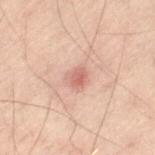| key | value |
|---|---|
| notes | no biopsy performed (imaged during a skin exam) |
| image | ~15 mm crop, total-body skin-cancer survey |
| illumination | cross-polarized illumination |
| subject | male, in their 40s |
| location | the left thigh |
| image-analysis metrics | a footprint of about 4.5 mm², an outline eccentricity of about 0.6 (0 = round, 1 = elongated), and a shape-asymmetry score of about 0.15 (0 = symmetric); a lesion color around L≈50 a*≈20 b*≈22 in CIELAB; peripheral color asymmetry of about 1 |
| diameter | ≈2.5 mm |